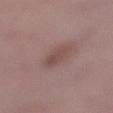follow-up: no biopsy performed (imaged during a skin exam) | lighting: white-light | automated lesion analysis: an outline eccentricity of about 0.75 (0 = round, 1 = elongated) and a symmetry-axis asymmetry near 0.15; a border-irregularity index near 2/10 and a within-lesion color-variation index near 2/10; a nevus-likeness score of about 65/100 and lesion-presence confidence of about 100/100 | location: the left lower leg | subject: female, roughly 45 years of age | diameter: ≈3 mm | image source: 15 mm crop, total-body photography.